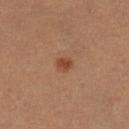Q: Was a biopsy performed?
A: imaged on a skin check; not biopsied
Q: What is the anatomic site?
A: the right thigh
Q: How was the tile lit?
A: cross-polarized illumination
Q: What kind of image is this?
A: total-body-photography crop, ~15 mm field of view
Q: Patient demographics?
A: female, aged around 30
Q: Lesion size?
A: ~2 mm (longest diameter)